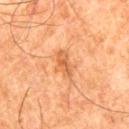workup — no biopsy performed (imaged during a skin exam)
acquisition — 15 mm crop, total-body photography
automated lesion analysis — a footprint of about 5 mm² and a symmetry-axis asymmetry near 0.35; about 8 CIELAB-L* units darker than the surrounding skin and a lesion-to-skin contrast of about 6 (normalized; higher = more distinct); a color-variation rating of about 3/10 and radial color variation of about 1; a detector confidence of about 100 out of 100 that the crop contains a lesion
lighting — cross-polarized
lesion size — about 3.5 mm
subject — male, aged 78 to 82
anatomic site — the right thigh